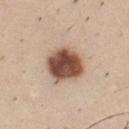Impression:
Part of a total-body skin-imaging series; this lesion was reviewed on a skin check and was not flagged for biopsy.
Image and clinical context:
Captured under white-light illumination. About 4.5 mm across. The lesion is on the chest. Cropped from a total-body skin-imaging series; the visible field is about 15 mm. A male patient, in their mid- to late 30s.